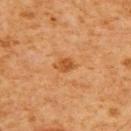| feature | finding |
|---|---|
| notes | catalogued during a skin exam; not biopsied |
| image | 15 mm crop, total-body photography |
| tile lighting | cross-polarized |
| size | ≈2.5 mm |
| anatomic site | the back |
| patient | male, aged approximately 60 |